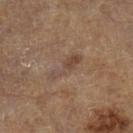{
  "biopsy_status": "not biopsied; imaged during a skin examination",
  "patient": {
    "sex": "male",
    "age_approx": "85+"
  },
  "image": {
    "source": "total-body photography crop",
    "field_of_view_mm": 15
  },
  "lighting": "cross-polarized",
  "site": "left lower leg"
}